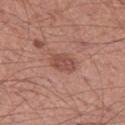<tbp_lesion>
<site>left thigh</site>
<automated_metrics>
  <eccentricity>0.75</eccentricity>
  <shape_asymmetry>0.2</shape_asymmetry>
  <color_variation_0_10>2.5</color_variation_0_10>
  <peripheral_color_asymmetry>1.0</peripheral_color_asymmetry>
  <nevus_likeness_0_100>40</nevus_likeness_0_100>
</automated_metrics>
<patient>
  <sex>male</sex>
  <age_approx>55</age_approx>
</patient>
<lesion_size>
  <long_diameter_mm_approx>3.0</long_diameter_mm_approx>
</lesion_size>
<image>
  <source>total-body photography crop</source>
  <field_of_view_mm>15</field_of_view_mm>
</image>
</tbp_lesion>